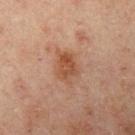Recorded during total-body skin imaging; not selected for excision or biopsy. A 15 mm close-up extracted from a 3D total-body photography capture. A male patient, approximately 45 years of age. The lesion is located on the left upper arm.